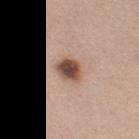{
  "biopsy_status": "not biopsied; imaged during a skin examination",
  "site": "left thigh",
  "lighting": "white-light",
  "lesion_size": {
    "long_diameter_mm_approx": 3.0
  },
  "patient": {
    "sex": "female",
    "age_approx": 40
  },
  "image": {
    "source": "total-body photography crop",
    "field_of_view_mm": 15
  }
}